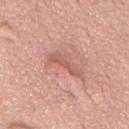Q: Was a biopsy performed?
A: total-body-photography surveillance lesion; no biopsy
Q: How large is the lesion?
A: ~4 mm (longest diameter)
Q: What did automated image analysis measure?
A: an average lesion color of about L≈58 a*≈27 b*≈28 (CIELAB) and a lesion–skin lightness drop of about 9; a border-irregularity index near 6.5/10, a color-variation rating of about 0/10, and peripheral color asymmetry of about 0
Q: What are the patient's age and sex?
A: male, approximately 75 years of age
Q: Illumination type?
A: white-light
Q: What is the anatomic site?
A: the back
Q: What is the imaging modality?
A: 15 mm crop, total-body photography